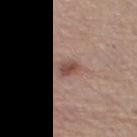Captured under white-light illumination. The recorded lesion diameter is about 3 mm. Located on the right thigh. The lesion-visualizer software estimated border irregularity of about 2 on a 0–10 scale and radial color variation of about 1. A region of skin cropped from a whole-body photographic capture, roughly 15 mm wide. The subject is a female in their mid- to late 60s.